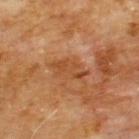Impression:
Recorded during total-body skin imaging; not selected for excision or biopsy.